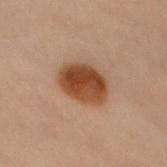Assessment:
This lesion was catalogued during total-body skin photography and was not selected for biopsy.
Clinical summary:
A female patient, aged approximately 60. A close-up tile cropped from a whole-body skin photograph, about 15 mm across. This is a cross-polarized tile. The lesion is on the back.